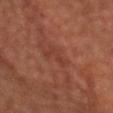follow-up — imaged on a skin check; not biopsied
tile lighting — cross-polarized illumination
lesion size — ~3.5 mm (longest diameter)
subject — male, in their 70s
image source — total-body-photography crop, ~15 mm field of view
image-analysis metrics — a lesion area of about 4 mm² and an eccentricity of roughly 0.95; a mean CIELAB color near L≈28 a*≈20 b*≈22 and about 4 CIELAB-L* units darker than the surrounding skin; a border-irregularity index near 6.5/10 and radial color variation of about 0; a nevus-likeness score of about 0/100 and a lesion-detection confidence of about 60/100
site — the head or neck